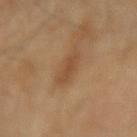* biopsy status: catalogued during a skin exam; not biopsied
* image: total-body-photography crop, ~15 mm field of view
* body site: the right forearm
* patient: female, aged 58–62